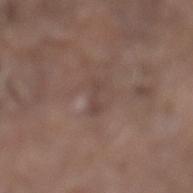* workup: imaged on a skin check; not biopsied
* automated metrics: a shape-asymmetry score of about 0.45 (0 = symmetric); a lesion color around L≈43 a*≈16 b*≈21 in CIELAB and a normalized border contrast of about 5; a border-irregularity rating of about 5.5/10, internal color variation of about 0 on a 0–10 scale, and radial color variation of about 0
* illumination: white-light
* location: the leg
* lesion size: ≈3 mm
* image source: total-body-photography crop, ~15 mm field of view
* subject: male, aged 78–82